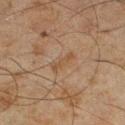Assessment: This lesion was catalogued during total-body skin photography and was not selected for biopsy. Image and clinical context: Approximately 3 mm at its widest. This is a cross-polarized tile. A region of skin cropped from a whole-body photographic capture, roughly 15 mm wide. An algorithmic analysis of the crop reported a lesion area of about 4.5 mm², an eccentricity of roughly 0.9, and a symmetry-axis asymmetry near 0.3. The analysis additionally found a mean CIELAB color near L≈41 a*≈14 b*≈27, roughly 5 lightness units darker than nearby skin, and a normalized border contrast of about 5. Located on the right lower leg. A male subject, in their mid- to late 40s.